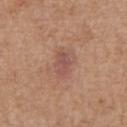<tbp_lesion>
  <automated_metrics>
    <vs_skin_darker_L>8.0</vs_skin_darker_L>
    <vs_skin_contrast_norm>6.0</vs_skin_contrast_norm>
  </automated_metrics>
  <lesion_size>
    <long_diameter_mm_approx>2.5</long_diameter_mm_approx>
  </lesion_size>
  <patient>
    <sex>male</sex>
    <age_approx>70</age_approx>
  </patient>
  <lighting>white-light</lighting>
  <image>
    <source>total-body photography crop</source>
    <field_of_view_mm>15</field_of_view_mm>
  </image>
  <site>chest</site>
</tbp_lesion>